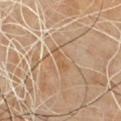Clinical impression:
Imaged during a routine full-body skin examination; the lesion was not biopsied and no histopathology is available.
Image and clinical context:
The lesion is located on the back. Longest diameter approximately 3 mm. The tile uses cross-polarized illumination. The subject is a male roughly 60 years of age. Cropped from a whole-body photographic skin survey; the tile spans about 15 mm.